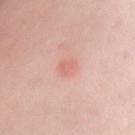The lesion was tiled from a total-body skin photograph and was not biopsied. A lesion tile, about 15 mm wide, cut from a 3D total-body photograph. Captured under white-light illumination. The lesion is located on the chest. The patient is a female approximately 50 years of age.